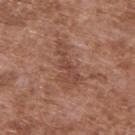<tbp_lesion>
<image>
  <source>total-body photography crop</source>
  <field_of_view_mm>15</field_of_view_mm>
</image>
<patient>
  <sex>male</sex>
  <age_approx>75</age_approx>
</patient>
<lighting>white-light</lighting>
<automated_metrics>
  <area_mm2_approx>8.5</area_mm2_approx>
  <eccentricity>0.95</eccentricity>
  <shape_asymmetry>0.5</shape_asymmetry>
  <nevus_likeness_0_100>0</nevus_likeness_0_100>
  <lesion_detection_confidence_0_100>100</lesion_detection_confidence_0_100>
</automated_metrics>
<site>upper back</site>
</tbp_lesion>